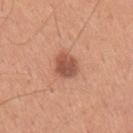Clinical impression: No biopsy was performed on this lesion — it was imaged during a full skin examination and was not determined to be concerning. Clinical summary: The recorded lesion diameter is about 3 mm. On the arm. A 15 mm close-up extracted from a 3D total-body photography capture. The patient is a male aged approximately 30. Automated image analysis of the tile measured a footprint of about 6.5 mm², an eccentricity of roughly 0.4, and two-axis asymmetry of about 0.2. The analysis additionally found a lesion color around L≈53 a*≈25 b*≈31 in CIELAB and a lesion–skin lightness drop of about 13. The software also gave an automated nevus-likeness rating near 80 out of 100 and a detector confidence of about 100 out of 100 that the crop contains a lesion.